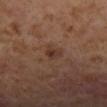Case summary:
• follow-up: catalogued during a skin exam; not biopsied
• subject: female, in their mid-50s
• image: ~15 mm tile from a whole-body skin photo
• lighting: cross-polarized illumination
• anatomic site: the left lower leg
• automated lesion analysis: a border-irregularity rating of about 2.5/10, internal color variation of about 5 on a 0–10 scale, and a peripheral color-asymmetry measure near 1.5
• lesion diameter: ≈2.5 mm